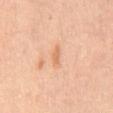Imaged during a routine full-body skin examination; the lesion was not biopsied and no histopathology is available. The total-body-photography lesion software estimated a lesion area of about 2 mm², a shape eccentricity near 0.9, and a symmetry-axis asymmetry near 0.3. It also reported a mean CIELAB color near L≈65 a*≈23 b*≈36 and a normalized border contrast of about 5.5. The software also gave a lesion-detection confidence of about 100/100. This image is a 15 mm lesion crop taken from a total-body photograph. The tile uses cross-polarized illumination. A male subject, about 60 years old. From the mid back. Approximately 2.5 mm at its widest.